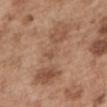Imaged during a routine full-body skin examination; the lesion was not biopsied and no histopathology is available. A male patient, aged 53 to 57. The lesion is located on the left upper arm. Imaged with white-light lighting. Approximately 9 mm at its widest. Automated tile analysis of the lesion measured a shape eccentricity near 0.95 and a shape-asymmetry score of about 0.4 (0 = symmetric). It also reported a nevus-likeness score of about 0/100 and a lesion-detection confidence of about 90/100. Cropped from a total-body skin-imaging series; the visible field is about 15 mm.